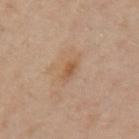biopsy_status: not biopsied; imaged during a skin examination
patient:
  sex: female
  age_approx: 40
site: left upper arm
image:
  source: total-body photography crop
  field_of_view_mm: 15